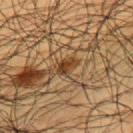| feature | finding |
|---|---|
| workup | no biopsy performed (imaged during a skin exam) |
| lighting | cross-polarized |
| site | the left upper arm |
| subject | male, aged approximately 60 |
| lesion diameter | ≈3 mm |
| imaging modality | ~15 mm tile from a whole-body skin photo |